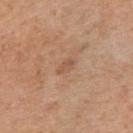{
  "lighting": "white-light",
  "image": {
    "source": "total-body photography crop",
    "field_of_view_mm": 15
  },
  "patient": {
    "sex": "male",
    "age_approx": 70
  },
  "site": "left upper arm",
  "lesion_size": {
    "long_diameter_mm_approx": 2.5
  }
}